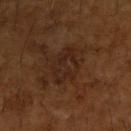Q: Was a biopsy performed?
A: total-body-photography surveillance lesion; no biopsy
Q: Patient demographics?
A: male, in their mid-60s
Q: Lesion size?
A: about 4.5 mm
Q: What lighting was used for the tile?
A: cross-polarized illumination
Q: Lesion location?
A: the right upper arm
Q: What kind of image is this?
A: ~15 mm crop, total-body skin-cancer survey
Q: Automated lesion metrics?
A: an average lesion color of about L≈24 a*≈17 b*≈25 (CIELAB), roughly 5 lightness units darker than nearby skin, and a normalized lesion–skin contrast near 6.5; border irregularity of about 3 on a 0–10 scale, a within-lesion color-variation index near 3.5/10, and radial color variation of about 1; a detector confidence of about 100 out of 100 that the crop contains a lesion